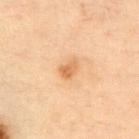| key | value |
|---|---|
| follow-up | catalogued during a skin exam; not biopsied |
| location | the chest |
| illumination | cross-polarized |
| subject | male, aged 53–57 |
| image source | ~15 mm crop, total-body skin-cancer survey |
| size | ~2.5 mm (longest diameter) |